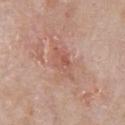workup: imaged on a skin check; not biopsied | anatomic site: the chest | automated lesion analysis: about 8 CIELAB-L* units darker than the surrounding skin; a border-irregularity index near 3/10 and radial color variation of about 1; a nevus-likeness score of about 0/100 | image source: ~15 mm crop, total-body skin-cancer survey | patient: male, roughly 65 years of age.